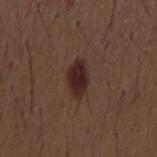<tbp_lesion>
<biopsy_status>not biopsied; imaged during a skin examination</biopsy_status>
</tbp_lesion>